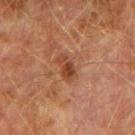The lesion was tiled from a total-body skin photograph and was not biopsied. A 15 mm close-up extracted from a 3D total-body photography capture. The lesion is located on the right upper arm. A male patient, approximately 75 years of age.